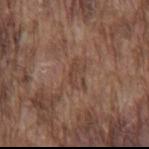Part of a total-body skin-imaging series; this lesion was reviewed on a skin check and was not flagged for biopsy. On the mid back. A close-up tile cropped from a whole-body skin photograph, about 15 mm across. The lesion's longest dimension is about 2.5 mm. The subject is a male aged around 75. This is a white-light tile.